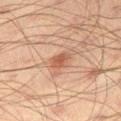The lesion was photographed on a routine skin check and not biopsied; there is no pathology result.
A male patient, roughly 45 years of age.
Cropped from a total-body skin-imaging series; the visible field is about 15 mm.
About 3 mm across.
From the leg.
Imaged with cross-polarized lighting.